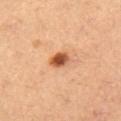Q: Was this lesion biopsied?
A: imaged on a skin check; not biopsied
Q: How was this image acquired?
A: ~15 mm crop, total-body skin-cancer survey
Q: What is the anatomic site?
A: the left thigh
Q: Who is the patient?
A: female, approximately 35 years of age
Q: Illumination type?
A: cross-polarized
Q: What did automated image analysis measure?
A: a lesion area of about 4.5 mm², an outline eccentricity of about 0.6 (0 = round, 1 = elongated), and two-axis asymmetry of about 0.2; a mean CIELAB color near L≈55 a*≈27 b*≈38 and about 16 CIELAB-L* units darker than the surrounding skin; a nevus-likeness score of about 100/100 and a detector confidence of about 100 out of 100 that the crop contains a lesion
Q: How large is the lesion?
A: ≈2.5 mm